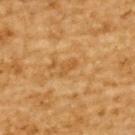workup = imaged on a skin check; not biopsied
size = ≈2.5 mm
image = total-body-photography crop, ~15 mm field of view
body site = the upper back
tile lighting = cross-polarized
patient = male, approximately 85 years of age or older
image-analysis metrics = a within-lesion color-variation index near 0/10 and peripheral color asymmetry of about 0